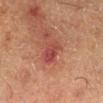<tbp_lesion>
  <image>
    <source>total-body photography crop</source>
    <field_of_view_mm>15</field_of_view_mm>
  </image>
  <patient>
    <sex>male</sex>
    <age_approx>60</age_approx>
  </patient>
  <site>right lower leg</site>
</tbp_lesion>